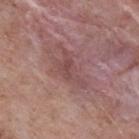acquisition: ~15 mm crop, total-body skin-cancer survey
lesion size: about 5 mm
site: the back
image-analysis metrics: a color-variation rating of about 3/10; a classifier nevus-likeness of about 0/100 and a detector confidence of about 70 out of 100 that the crop contains a lesion
illumination: white-light illumination
subject: male, about 70 years old